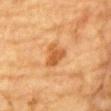The lesion was tiled from a total-body skin photograph and was not biopsied.
Automated image analysis of the tile measured a lesion area of about 6.5 mm² and a shape eccentricity near 0.65. It also reported an average lesion color of about L≈51 a*≈24 b*≈40 (CIELAB) and a normalized border contrast of about 7. And it measured border irregularity of about 3.5 on a 0–10 scale, internal color variation of about 2.5 on a 0–10 scale, and radial color variation of about 1.
Imaged with cross-polarized lighting.
The subject is a male aged approximately 85 or older.
On the front of the torso.
Measured at roughly 3.5 mm in maximum diameter.
A 15 mm close-up extracted from a 3D total-body photography capture.